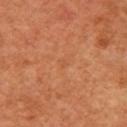Impression:
No biopsy was performed on this lesion — it was imaged during a full skin examination and was not determined to be concerning.
Clinical summary:
From the right upper arm. About 1 mm across. Cropped from a total-body skin-imaging series; the visible field is about 15 mm. The patient is a female approximately 65 years of age.